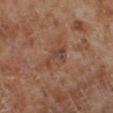* workup: total-body-photography surveillance lesion; no biopsy
* tile lighting: cross-polarized illumination
* subject: male, in their 70s
* site: the left lower leg
* acquisition: ~15 mm crop, total-body skin-cancer survey
* lesion size: ≈3.5 mm
* TBP lesion metrics: a lesion area of about 4.5 mm², an outline eccentricity of about 0.85 (0 = round, 1 = elongated), and two-axis asymmetry of about 0.55; a classifier nevus-likeness of about 0/100 and lesion-presence confidence of about 100/100